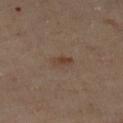| key | value |
|---|---|
| biopsy status | total-body-photography surveillance lesion; no biopsy |
| image source | ~15 mm crop, total-body skin-cancer survey |
| subject | female, approximately 60 years of age |
| location | the left lower leg |
| illumination | cross-polarized |
| image-analysis metrics | a within-lesion color-variation index near 3.5/10 and a peripheral color-asymmetry measure near 1; a classifier nevus-likeness of about 15/100 and lesion-presence confidence of about 100/100 |